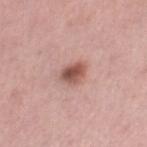No biopsy was performed on this lesion — it was imaged during a full skin examination and was not determined to be concerning.
The lesion-visualizer software estimated a border-irregularity index near 2/10, a within-lesion color-variation index near 6/10, and a peripheral color-asymmetry measure near 2. It also reported an automated nevus-likeness rating near 90 out of 100 and a detector confidence of about 100 out of 100 that the crop contains a lesion.
A 15 mm close-up extracted from a 3D total-body photography capture.
The patient is a female aged around 55.
Approximately 2.5 mm at its widest.
The tile uses white-light illumination.
From the right thigh.